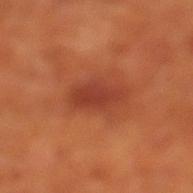Assessment:
No biopsy was performed on this lesion — it was imaged during a full skin examination and was not determined to be concerning.
Acquisition and patient details:
Cropped from a whole-body photographic skin survey; the tile spans about 15 mm. The lesion-visualizer software estimated a footprint of about 8.5 mm², a shape eccentricity near 0.8, and a symmetry-axis asymmetry near 0.2. The analysis additionally found border irregularity of about 2 on a 0–10 scale, internal color variation of about 2.5 on a 0–10 scale, and peripheral color asymmetry of about 0.5. On the left lower leg. The patient is a male aged 68–72.